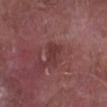The lesion was photographed on a routine skin check and not biopsied; there is no pathology result.
A roughly 15 mm field-of-view crop from a total-body skin photograph.
An algorithmic analysis of the crop reported an area of roughly 4 mm², an eccentricity of roughly 0.75, and two-axis asymmetry of about 0.35. The analysis additionally found an average lesion color of about L≈35 a*≈24 b*≈20 (CIELAB), about 7 CIELAB-L* units darker than the surrounding skin, and a lesion-to-skin contrast of about 6.5 (normalized; higher = more distinct). And it measured a border-irregularity index near 3.5/10, internal color variation of about 1 on a 0–10 scale, and a peripheral color-asymmetry measure near 0. It also reported a classifier nevus-likeness of about 0/100.
Imaged with white-light lighting.
A male subject, aged approximately 80.
Longest diameter approximately 2.5 mm.
From the leg.